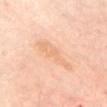A female patient, in their 60s.
A lesion tile, about 15 mm wide, cut from a 3D total-body photograph.
Located on the chest.